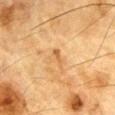Assessment: Part of a total-body skin-imaging series; this lesion was reviewed on a skin check and was not flagged for biopsy. Clinical summary: A 15 mm crop from a total-body photograph taken for skin-cancer surveillance. A male subject, in their mid- to late 80s or older. Located on the chest.